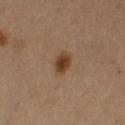workup = no biopsy performed (imaged during a skin exam)
acquisition = total-body-photography crop, ~15 mm field of view
patient = male, about 50 years old
location = the left arm
lighting = cross-polarized